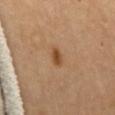<lesion>
<biopsy_status>not biopsied; imaged during a skin examination</biopsy_status>
<patient>
  <sex>female</sex>
  <age_approx>65</age_approx>
</patient>
<automated_metrics>
  <area_mm2_approx>3.0</area_mm2_approx>
  <eccentricity>0.85</eccentricity>
  <shape_asymmetry>0.25</shape_asymmetry>
  <cielab_L>46</cielab_L>
  <cielab_a>20</cielab_a>
  <cielab_b>35</cielab_b>
  <vs_skin_darker_L>10.0</vs_skin_darker_L>
  <vs_skin_contrast_norm>8.5</vs_skin_contrast_norm>
  <border_irregularity_0_10>2.5</border_irregularity_0_10>
  <color_variation_0_10>2.0</color_variation_0_10>
  <nevus_likeness_0_100>90</nevus_likeness_0_100>
  <lesion_detection_confidence_0_100>100</lesion_detection_confidence_0_100>
</automated_metrics>
<image>
  <source>total-body photography crop</source>
  <field_of_view_mm>15</field_of_view_mm>
</image>
<lesion_size>
  <long_diameter_mm_approx>3.0</long_diameter_mm_approx>
</lesion_size>
<site>front of the torso</site>
</lesion>